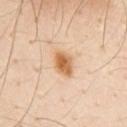The lesion was tiled from a total-body skin photograph and was not biopsied. The lesion is located on the chest. Captured under cross-polarized illumination. The patient is a male aged 28–32. Longest diameter approximately 3.5 mm. Cropped from a total-body skin-imaging series; the visible field is about 15 mm.